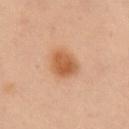<lesion>
  <image>
    <source>total-body photography crop</source>
    <field_of_view_mm>15</field_of_view_mm>
  </image>
  <lesion_size>
    <long_diameter_mm_approx>3.5</long_diameter_mm_approx>
  </lesion_size>
  <site>right upper arm</site>
  <patient>
    <sex>male</sex>
    <age_approx>55</age_approx>
  </patient>
</lesion>